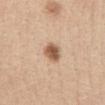notes: no biopsy performed (imaged during a skin exam) | patient: female, aged 33 to 37 | body site: the chest | imaging modality: total-body-photography crop, ~15 mm field of view | lighting: white-light | lesion diameter: ~2.5 mm (longest diameter).